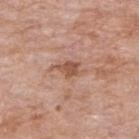biopsy_status: not biopsied; imaged during a skin examination
site: right upper arm
lighting: white-light
automated_metrics:
  area_mm2_approx: 5.0
  eccentricity: 0.85
  shape_asymmetry: 0.45
  cielab_L: 54
  cielab_a: 22
  cielab_b: 29
  vs_skin_contrast_norm: 7.0
  border_irregularity_0_10: 5.0
  color_variation_0_10: 2.0
  peripheral_color_asymmetry: 0.5
  nevus_likeness_0_100: 0
  lesion_detection_confidence_0_100: 100
image:
  source: total-body photography crop
  field_of_view_mm: 15
patient:
  sex: female
  age_approx: 70